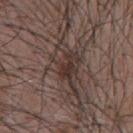A roughly 15 mm field-of-view crop from a total-body skin photograph. A male patient, roughly 55 years of age. An algorithmic analysis of the crop reported a footprint of about 13 mm², a shape eccentricity near 0.85, and a shape-asymmetry score of about 0.3 (0 = symmetric). Measured at roughly 6 mm in maximum diameter. Captured under white-light illumination. On the chest.